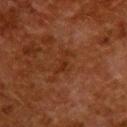workup: catalogued during a skin exam; not biopsied | imaging modality: 15 mm crop, total-body photography | lesion diameter: about 3 mm | automated metrics: a lesion area of about 3 mm², an outline eccentricity of about 0.9 (0 = round, 1 = elongated), and a symmetry-axis asymmetry near 0.5; a lesion color around L≈24 a*≈21 b*≈28 in CIELAB, about 4 CIELAB-L* units darker than the surrounding skin, and a normalized border contrast of about 5.5; border irregularity of about 6.5 on a 0–10 scale, internal color variation of about 0 on a 0–10 scale, and a peripheral color-asymmetry measure near 0; a classifier nevus-likeness of about 0/100 and a lesion-detection confidence of about 100/100 | tile lighting: cross-polarized | subject: female, aged 48–52 | site: the upper back.